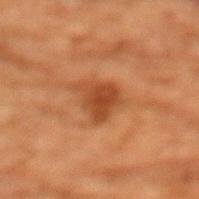Part of a total-body skin-imaging series; this lesion was reviewed on a skin check and was not flagged for biopsy. A female patient, roughly 80 years of age. Measured at roughly 4 mm in maximum diameter. On the chest. A close-up tile cropped from a whole-body skin photograph, about 15 mm across.